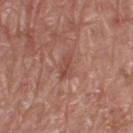Q: Was a biopsy performed?
A: catalogued during a skin exam; not biopsied
Q: What kind of image is this?
A: total-body-photography crop, ~15 mm field of view
Q: Patient demographics?
A: male, aged approximately 70
Q: What lighting was used for the tile?
A: white-light illumination
Q: What is the anatomic site?
A: the right thigh
Q: Lesion size?
A: ≈3 mm